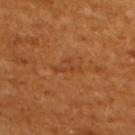notes = total-body-photography surveillance lesion; no biopsy
acquisition = ~15 mm crop, total-body skin-cancer survey
site = the upper back
patient = female, aged approximately 55